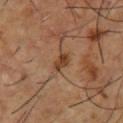Q: Was a biopsy performed?
A: no biopsy performed (imaged during a skin exam)
Q: How large is the lesion?
A: ≈2.5 mm
Q: Where on the body is the lesion?
A: the chest
Q: What are the patient's age and sex?
A: male, aged approximately 55
Q: How was the tile lit?
A: cross-polarized illumination
Q: Automated lesion metrics?
A: a lesion color around L≈40 a*≈20 b*≈33 in CIELAB, a lesion–skin lightness drop of about 10, and a lesion-to-skin contrast of about 8.5 (normalized; higher = more distinct); a border-irregularity rating of about 2.5/10, internal color variation of about 3 on a 0–10 scale, and a peripheral color-asymmetry measure near 1; a nevus-likeness score of about 70/100 and lesion-presence confidence of about 100/100
Q: What kind of image is this?
A: total-body-photography crop, ~15 mm field of view